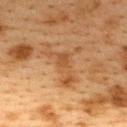Notes:
* size: ~6 mm (longest diameter)
* patient: female, aged 38–42
* image: total-body-photography crop, ~15 mm field of view
* anatomic site: the upper back
* TBP lesion metrics: a lesion area of about 10 mm², an outline eccentricity of about 0.75 (0 = round, 1 = elongated), and two-axis asymmetry of about 0.7